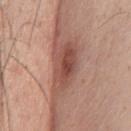  biopsy_status: not biopsied; imaged during a skin examination
  image:
    source: total-body photography crop
    field_of_view_mm: 15
  lesion_size:
    long_diameter_mm_approx: 6.0
  patient:
    sex: male
    age_approx: 60
  site: chest
  lighting: white-light